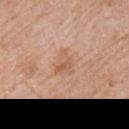| feature | finding |
|---|---|
| biopsy status | catalogued during a skin exam; not biopsied |
| location | the right upper arm |
| image | ~15 mm tile from a whole-body skin photo |
| patient | male, roughly 50 years of age |
| lesion diameter | about 3 mm |
| tile lighting | white-light illumination |